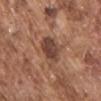workup: no biopsy performed (imaged during a skin exam)
size: ~4 mm (longest diameter)
tile lighting: white-light illumination
automated metrics: an area of roughly 10 mm², a shape eccentricity near 0.45, and two-axis asymmetry of about 0.25; a mean CIELAB color near L≈43 a*≈20 b*≈26, about 11 CIELAB-L* units darker than the surrounding skin, and a normalized lesion–skin contrast near 9; a within-lesion color-variation index near 4.5/10
imaging modality: 15 mm crop, total-body photography
anatomic site: the chest
subject: male, aged approximately 75